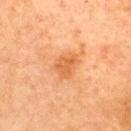| field | value |
|---|---|
| notes | imaged on a skin check; not biopsied |
| subject | female, aged 58 to 62 |
| image | 15 mm crop, total-body photography |
| location | the upper back |
| TBP lesion metrics | border irregularity of about 3.5 on a 0–10 scale, internal color variation of about 2 on a 0–10 scale, and peripheral color asymmetry of about 0.5 |
| size | ~3 mm (longest diameter) |